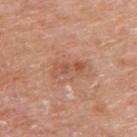| field | value |
|---|---|
| follow-up | imaged on a skin check; not biopsied |
| lesion size | about 4 mm |
| acquisition | ~15 mm tile from a whole-body skin photo |
| body site | the right upper arm |
| patient | male, aged 78–82 |